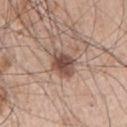patient: male, aged approximately 65 | imaging modality: ~15 mm crop, total-body skin-cancer survey | site: the right upper arm.